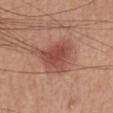notes=catalogued during a skin exam; not biopsied | imaging modality=15 mm crop, total-body photography | anatomic site=the front of the torso | patient=male, aged around 65 | tile lighting=white-light illumination | image-analysis metrics=an automated nevus-likeness rating near 95 out of 100 and a lesion-detection confidence of about 100/100 | diameter=≈4.5 mm.